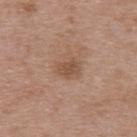  biopsy_status: not biopsied; imaged during a skin examination
  site: upper back
  automated_metrics:
    area_mm2_approx: 4.0
    eccentricity: 0.75
    shape_asymmetry: 0.2
    border_irregularity_0_10: 2.0
    color_variation_0_10: 2.0
    peripheral_color_asymmetry: 0.5
  lighting: white-light
  image:
    source: total-body photography crop
    field_of_view_mm: 15
  lesion_size:
    long_diameter_mm_approx: 2.5
  patient:
    sex: male
    age_approx: 50